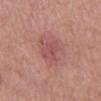Clinical impression: Part of a total-body skin-imaging series; this lesion was reviewed on a skin check and was not flagged for biopsy. Acquisition and patient details: Located on the mid back. Automated image analysis of the tile measured a mean CIELAB color near L≈52 a*≈27 b*≈23 and roughly 7 lightness units darker than nearby skin. The analysis additionally found a classifier nevus-likeness of about 0/100 and a lesion-detection confidence of about 100/100. The lesion's longest dimension is about 4 mm. This is a white-light tile. A lesion tile, about 15 mm wide, cut from a 3D total-body photograph. The subject is a male approximately 60 years of age.